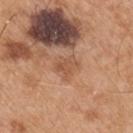Impression:
No biopsy was performed on this lesion — it was imaged during a full skin examination and was not determined to be concerning.
Acquisition and patient details:
The lesion's longest dimension is about 3 mm. On the right upper arm. Imaged with white-light lighting. A 15 mm close-up tile from a total-body photography series done for melanoma screening. A male patient, approximately 55 years of age.